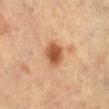The lesion was tiled from a total-body skin photograph and was not biopsied. A 15 mm close-up tile from a total-body photography series done for melanoma screening. The lesion is on the left lower leg. A female patient, in their mid-50s.